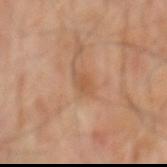Case summary:
– lighting: cross-polarized
– anatomic site: the mid back
– patient: male, about 70 years old
– image: 15 mm crop, total-body photography